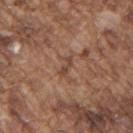Case summary:
– notes · no biopsy performed (imaged during a skin exam)
– location · the upper back
– image source · total-body-photography crop, ~15 mm field of view
– subject · male, aged approximately 75